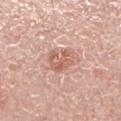Findings:
- notes — imaged on a skin check; not biopsied
- tile lighting — white-light illumination
- lesion diameter — ≈3 mm
- subject — male, in their 70s
- automated metrics — roughly 9 lightness units darker than nearby skin and a lesion-to-skin contrast of about 6.5 (normalized; higher = more distinct); an automated nevus-likeness rating near 20 out of 100 and a lesion-detection confidence of about 100/100
- image — ~15 mm crop, total-body skin-cancer survey
- body site — the left lower leg